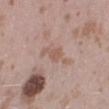Captured during whole-body skin photography for melanoma surveillance; the lesion was not biopsied. Located on the right lower leg. A close-up tile cropped from a whole-body skin photograph, about 15 mm across. Approximately 4.5 mm at its widest. Automated tile analysis of the lesion measured a shape-asymmetry score of about 0.4 (0 = symmetric). It also reported a mean CIELAB color near L≈57 a*≈17 b*≈26. The subject is a female approximately 25 years of age. The tile uses white-light illumination.